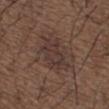workup: no biopsy performed (imaged during a skin exam)
anatomic site: the upper back
lesion size: about 5.5 mm
image source: 15 mm crop, total-body photography
subject: male, roughly 50 years of age
illumination: white-light